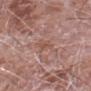| key | value |
|---|---|
| biopsy status | total-body-photography surveillance lesion; no biopsy |
| imaging modality | ~15 mm tile from a whole-body skin photo |
| patient | male, aged approximately 75 |
| size | about 2.5 mm |
| location | the right forearm |
| automated lesion analysis | a lesion area of about 3 mm², an outline eccentricity of about 0.85 (0 = round, 1 = elongated), and a shape-asymmetry score of about 0.35 (0 = symmetric); a lesion–skin lightness drop of about 6 and a normalized border contrast of about 5.5 |